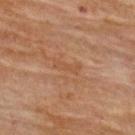Findings:
– biopsy status — total-body-photography surveillance lesion; no biopsy
– subject — female, aged 78 to 82
– anatomic site — the upper back
– image source — 15 mm crop, total-body photography
– tile lighting — cross-polarized
– TBP lesion metrics — a footprint of about 2.5 mm², an outline eccentricity of about 0.95 (0 = round, 1 = elongated), and a symmetry-axis asymmetry near 0.5; a color-variation rating of about 0/10 and a peripheral color-asymmetry measure near 0; a lesion-detection confidence of about 100/100
– lesion diameter — about 3 mm